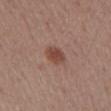Assessment:
This lesion was catalogued during total-body skin photography and was not selected for biopsy.
Acquisition and patient details:
A roughly 15 mm field-of-view crop from a total-body skin photograph. The recorded lesion diameter is about 3 mm. Captured under white-light illumination. A male subject, roughly 55 years of age. From the abdomen.